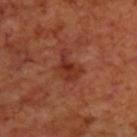This is a cross-polarized tile.
Cropped from a total-body skin-imaging series; the visible field is about 15 mm.
About 3.5 mm across.
The subject is a male aged 68 to 72.
Located on the upper back.
An algorithmic analysis of the crop reported a footprint of about 6 mm², a shape eccentricity near 0.75, and two-axis asymmetry of about 0.45. It also reported a border-irregularity index near 5/10 and peripheral color asymmetry of about 1. The analysis additionally found a classifier nevus-likeness of about 0/100 and a detector confidence of about 100 out of 100 that the crop contains a lesion.